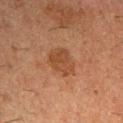Recorded during total-body skin imaging; not selected for excision or biopsy.